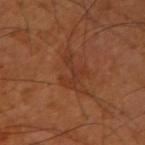| feature | finding |
|---|---|
| follow-up | catalogued during a skin exam; not biopsied |
| automated metrics | an area of roughly 5 mm², an eccentricity of roughly 0.75, and a symmetry-axis asymmetry near 0.8; a lesion color around L≈34 a*≈24 b*≈31 in CIELAB and a normalized border contrast of about 5; a border-irregularity rating of about 9/10 and peripheral color asymmetry of about 0 |
| patient | male, aged approximately 60 |
| body site | the right upper arm |
| tile lighting | cross-polarized illumination |
| image source | 15 mm crop, total-body photography |
| lesion diameter | ~4 mm (longest diameter) |